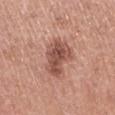The lesion was photographed on a routine skin check and not biopsied; there is no pathology result.
The tile uses white-light illumination.
Automated tile analysis of the lesion measured a lesion area of about 12 mm², a shape eccentricity near 0.85, and a symmetry-axis asymmetry near 0.3. The software also gave about 13 CIELAB-L* units darker than the surrounding skin and a lesion-to-skin contrast of about 9 (normalized; higher = more distinct). The software also gave an automated nevus-likeness rating near 60 out of 100.
A lesion tile, about 15 mm wide, cut from a 3D total-body photograph.
On the right upper arm.
The patient is a female in their mid-60s.
Measured at roughly 5.5 mm in maximum diameter.